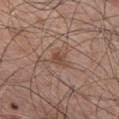Case summary:
- biopsy status: imaged on a skin check; not biopsied
- patient: male, in their 50s
- acquisition: ~15 mm crop, total-body skin-cancer survey
- anatomic site: the chest